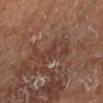Q: Is there a histopathology result?
A: total-body-photography surveillance lesion; no biopsy
Q: How was the tile lit?
A: cross-polarized
Q: How was this image acquired?
A: total-body-photography crop, ~15 mm field of view
Q: What did automated image analysis measure?
A: a footprint of about 8.5 mm², an outline eccentricity of about 0.9 (0 = round, 1 = elongated), and a symmetry-axis asymmetry near 0.55; a mean CIELAB color near L≈38 a*≈21 b*≈25 and a lesion-to-skin contrast of about 5.5 (normalized; higher = more distinct); a nevus-likeness score of about 0/100 and a lesion-detection confidence of about 70/100
Q: Who is the patient?
A: male, aged around 65
Q: Lesion location?
A: the right lower leg